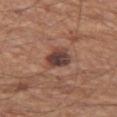Context: A 15 mm crop from a total-body photograph taken for skin-cancer surveillance. The total-body-photography lesion software estimated an automated nevus-likeness rating near 5 out of 100. The tile uses white-light illumination. From the left thigh. A male patient aged around 65. Measured at roughly 3.5 mm in maximum diameter.